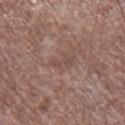  biopsy_status: not biopsied; imaged during a skin examination
  site: right lower leg
  image:
    source: total-body photography crop
    field_of_view_mm: 15
  lighting: white-light
  lesion_size:
    long_diameter_mm_approx: 3.0
  automated_metrics:
    cielab_L: 47
    cielab_a: 18
    cielab_b: 23
    vs_skin_darker_L: 6.0
    vs_skin_contrast_norm: 5.0
  patient:
    sex: male
    age_approx: 70